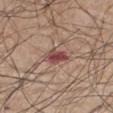{"biopsy_status": "not biopsied; imaged during a skin examination", "patient": {"sex": "male", "age_approx": 75}, "lighting": "white-light", "lesion_size": {"long_diameter_mm_approx": 3.0}, "image": {"source": "total-body photography crop", "field_of_view_mm": 15}, "site": "right thigh"}